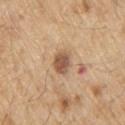notes: imaged on a skin check; not biopsied | tile lighting: white-light | acquisition: ~15 mm tile from a whole-body skin photo | subject: male, in their 70s | site: the upper back.